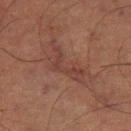The lesion is on the left thigh. A male patient, aged 63–67. Measured at roughly 6 mm in maximum diameter. Cropped from a total-body skin-imaging series; the visible field is about 15 mm.